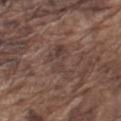Cropped from a total-body skin-imaging series; the visible field is about 15 mm.
The patient is a male aged 73–77.
The total-body-photography lesion software estimated a lesion area of about 7.5 mm², a shape eccentricity near 0.8, and a symmetry-axis asymmetry near 0.5. It also reported a border-irregularity index near 8/10, a within-lesion color-variation index near 4/10, and radial color variation of about 1.5.
The lesion is located on the left upper arm.
The tile uses white-light illumination.